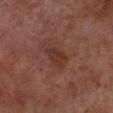Q: Is there a histopathology result?
A: no biopsy performed (imaged during a skin exam)
Q: What kind of image is this?
A: ~15 mm tile from a whole-body skin photo
Q: What did automated image analysis measure?
A: an area of roughly 5 mm² and a shape-asymmetry score of about 0.3 (0 = symmetric); a lesion color around L≈25 a*≈18 b*≈20 in CIELAB; internal color variation of about 2 on a 0–10 scale and a peripheral color-asymmetry measure near 0.5
Q: What are the patient's age and sex?
A: male, aged 68–72
Q: What is the anatomic site?
A: the right lower leg